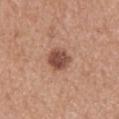This lesion was catalogued during total-body skin photography and was not selected for biopsy. A roughly 15 mm field-of-view crop from a total-body skin photograph. On the back. The subject is a female in their mid-30s. Imaged with white-light lighting. Approximately 3 mm at its widest. The lesion-visualizer software estimated an outline eccentricity of about 0.3 (0 = round, 1 = elongated) and a shape-asymmetry score of about 0.2 (0 = symmetric). It also reported a mean CIELAB color near L≈49 a*≈23 b*≈27 and about 14 CIELAB-L* units darker than the surrounding skin. And it measured border irregularity of about 2 on a 0–10 scale, a within-lesion color-variation index near 3.5/10, and a peripheral color-asymmetry measure near 1. It also reported a nevus-likeness score of about 70/100 and lesion-presence confidence of about 100/100.